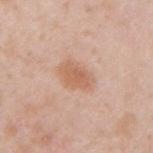Imaged during a routine full-body skin examination; the lesion was not biopsied and no histopathology is available.
The lesion is located on the right upper arm.
A male patient, roughly 35 years of age.
The lesion-visualizer software estimated a footprint of about 7 mm², an outline eccentricity of about 0.8 (0 = round, 1 = elongated), and a symmetry-axis asymmetry near 0.25. The analysis additionally found an average lesion color of about L≈61 a*≈22 b*≈32 (CIELAB), roughly 9 lightness units darker than nearby skin, and a lesion-to-skin contrast of about 6.5 (normalized; higher = more distinct).
A 15 mm close-up tile from a total-body photography series done for melanoma screening.
This is a white-light tile.